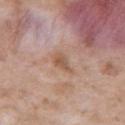Case summary:
– lesion size — ~3 mm (longest diameter)
– TBP lesion metrics — two-axis asymmetry of about 0.35; a peripheral color-asymmetry measure near 0.5; an automated nevus-likeness rating near 0 out of 100 and a detector confidence of about 100 out of 100 that the crop contains a lesion
– subject — male, about 50 years old
– site — the right upper arm
– lighting — white-light
– imaging modality — 15 mm crop, total-body photography